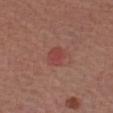The lesion was tiled from a total-body skin photograph and was not biopsied.
On the left forearm.
The subject is a male in their mid- to late 50s.
Automated tile analysis of the lesion measured an area of roughly 4.5 mm², an eccentricity of roughly 0.7, and two-axis asymmetry of about 0.25. And it measured an average lesion color of about L≈44 a*≈28 b*≈25 (CIELAB), a lesion–skin lightness drop of about 7, and a lesion-to-skin contrast of about 5.5 (normalized; higher = more distinct). It also reported internal color variation of about 2 on a 0–10 scale and radial color variation of about 1.
A close-up tile cropped from a whole-body skin photograph, about 15 mm across.